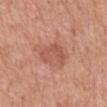A 15 mm close-up tile from a total-body photography series done for melanoma screening.
An algorithmic analysis of the crop reported a mean CIELAB color near L≈54 a*≈26 b*≈29. The software also gave an automated nevus-likeness rating near 40 out of 100 and lesion-presence confidence of about 100/100.
The lesion is on the back.
A male subject, aged approximately 70.